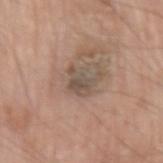Assessment:
No biopsy was performed on this lesion — it was imaged during a full skin examination and was not determined to be concerning.
Context:
A lesion tile, about 15 mm wide, cut from a 3D total-body photograph. Automated image analysis of the tile measured a lesion–skin lightness drop of about 9 and a lesion-to-skin contrast of about 6.5 (normalized; higher = more distinct). The software also gave a border-irregularity index near 7/10 and a within-lesion color-variation index near 3/10. It also reported an automated nevus-likeness rating near 0 out of 100 and a detector confidence of about 100 out of 100 that the crop contains a lesion. The lesion's longest dimension is about 3.5 mm. The patient is a male aged 73 to 77. The lesion is on the left upper arm. The tile uses white-light illumination.